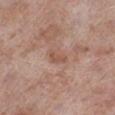Impression: The lesion was photographed on a routine skin check and not biopsied; there is no pathology result. Acquisition and patient details: From the right lower leg. The lesion-visualizer software estimated a mean CIELAB color near L≈53 a*≈21 b*≈27, roughly 7 lightness units darker than nearby skin, and a lesion-to-skin contrast of about 5.5 (normalized; higher = more distinct). And it measured an automated nevus-likeness rating near 0 out of 100. The tile uses white-light illumination. The subject is a male about 75 years old. A roughly 15 mm field-of-view crop from a total-body skin photograph. Approximately 2.5 mm at its widest.